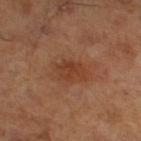{
  "biopsy_status": "not biopsied; imaged during a skin examination",
  "patient": {
    "sex": "male",
    "age_approx": 65
  },
  "lighting": "cross-polarized",
  "automated_metrics": {
    "border_irregularity_0_10": 2.5,
    "color_variation_0_10": 2.5,
    "peripheral_color_asymmetry": 1.0,
    "nevus_likeness_0_100": 75,
    "lesion_detection_confidence_0_100": 100
  },
  "image": {
    "source": "total-body photography crop",
    "field_of_view_mm": 15
  }
}